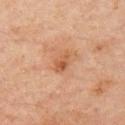Q: Is there a histopathology result?
A: imaged on a skin check; not biopsied
Q: What kind of image is this?
A: total-body-photography crop, ~15 mm field of view
Q: What is the anatomic site?
A: the arm
Q: Who is the patient?
A: male, aged approximately 45